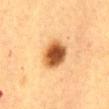The lesion was tiled from a total-body skin photograph and was not biopsied.